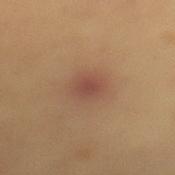No biopsy was performed on this lesion — it was imaged during a full skin examination and was not determined to be concerning. This is a cross-polarized tile. Automated tile analysis of the lesion measured a lesion area of about 5.5 mm², an outline eccentricity of about 0.55 (0 = round, 1 = elongated), and two-axis asymmetry of about 0.2. It also reported a lesion color around L≈45 a*≈21 b*≈26 in CIELAB, roughly 7 lightness units darker than nearby skin, and a normalized border contrast of about 6.5. And it measured a border-irregularity rating of about 2/10, a within-lesion color-variation index near 2.5/10, and a peripheral color-asymmetry measure near 0.5. The software also gave an automated nevus-likeness rating near 5 out of 100 and a lesion-detection confidence of about 100/100. A close-up tile cropped from a whole-body skin photograph, about 15 mm across. The patient is a male aged approximately 55. The lesion is on the mid back. Longest diameter approximately 3 mm.